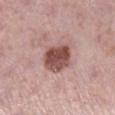Case summary:
- patient · female, aged 38 to 42
- location · the right lower leg
- acquisition · 15 mm crop, total-body photography